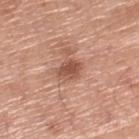– biopsy status — no biopsy performed (imaged during a skin exam)
– lighting — white-light
– automated lesion analysis — an area of roughly 5.5 mm², an eccentricity of roughly 0.6, and two-axis asymmetry of about 0.25; a border-irregularity index near 2/10 and peripheral color asymmetry of about 1; a classifier nevus-likeness of about 80/100 and a lesion-detection confidence of about 100/100
– lesion size — ≈3 mm
– image — ~15 mm crop, total-body skin-cancer survey
– anatomic site — the right lower leg
– subject — male, roughly 80 years of age